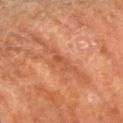Q: Was this lesion biopsied?
A: catalogued during a skin exam; not biopsied